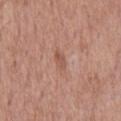biopsy_status: not biopsied; imaged during a skin examination
image:
  source: total-body photography crop
  field_of_view_mm: 15
lesion_size:
  long_diameter_mm_approx: 2.5
lighting: white-light
patient:
  sex: male
  age_approx: 60
site: back